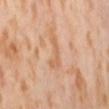Clinical impression:
Part of a total-body skin-imaging series; this lesion was reviewed on a skin check and was not flagged for biopsy.
Context:
Located on the back. Captured under cross-polarized illumination. A female subject, in their mid-50s. Automated image analysis of the tile measured a footprint of about 5 mm² and a shape-asymmetry score of about 0.55 (0 = symmetric). It also reported a border-irregularity rating of about 6.5/10 and a within-lesion color-variation index near 0.5/10. A 15 mm crop from a total-body photograph taken for skin-cancer surveillance.